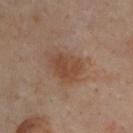Q: Was this lesion biopsied?
A: no biopsy performed (imaged during a skin exam)
Q: Automated lesion metrics?
A: a footprint of about 9 mm² and an outline eccentricity of about 0.75 (0 = round, 1 = elongated); an average lesion color of about L≈43 a*≈19 b*≈28 (CIELAB) and roughly 8 lightness units darker than nearby skin; a classifier nevus-likeness of about 70/100 and lesion-presence confidence of about 100/100
Q: Where on the body is the lesion?
A: the upper back
Q: What lighting was used for the tile?
A: cross-polarized illumination
Q: What are the patient's age and sex?
A: male, about 45 years old
Q: How was this image acquired?
A: ~15 mm tile from a whole-body skin photo